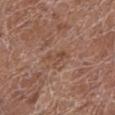Recorded during total-body skin imaging; not selected for excision or biopsy.
Approximately 2.5 mm at its widest.
The lesion-visualizer software estimated a mean CIELAB color near L≈47 a*≈20 b*≈28, about 7 CIELAB-L* units darker than the surrounding skin, and a normalized lesion–skin contrast near 5.5. The analysis additionally found an automated nevus-likeness rating near 0 out of 100 and a lesion-detection confidence of about 100/100.
The lesion is on the left lower leg.
Imaged with white-light lighting.
A lesion tile, about 15 mm wide, cut from a 3D total-body photograph.
The patient is a female in their 80s.